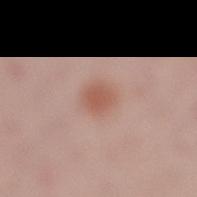follow-up = catalogued during a skin exam; not biopsied | location = the right lower leg | patient = female, approximately 25 years of age | lighting = white-light | lesion size = ~2.5 mm (longest diameter) | image source = ~15 mm crop, total-body skin-cancer survey.